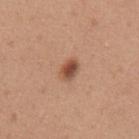| field | value |
|---|---|
| follow-up | total-body-photography surveillance lesion; no biopsy |
| illumination | white-light |
| subject | female, about 45 years old |
| image source | ~15 mm crop, total-body skin-cancer survey |
| lesion diameter | ~2.5 mm (longest diameter) |
| location | the arm |